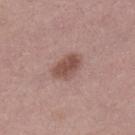Impression: No biopsy was performed on this lesion — it was imaged during a full skin examination and was not determined to be concerning. Clinical summary: A 15 mm close-up extracted from a 3D total-body photography capture. The lesion is located on the left lower leg. A female subject about 40 years old.